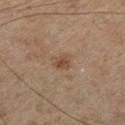No biopsy was performed on this lesion — it was imaged during a full skin examination and was not determined to be concerning. A 15 mm close-up extracted from a 3D total-body photography capture. The lesion is on the left lower leg. A male patient, aged approximately 70. Measured at roughly 2.5 mm in maximum diameter. Automated tile analysis of the lesion measured a lesion color around L≈35 a*≈13 b*≈23 in CIELAB, about 6 CIELAB-L* units darker than the surrounding skin, and a normalized border contrast of about 6. The analysis additionally found a nevus-likeness score of about 40/100. This is a cross-polarized tile.